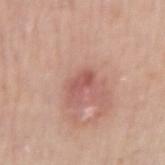Impression: No biopsy was performed on this lesion — it was imaged during a full skin examination and was not determined to be concerning. Image and clinical context: Longest diameter approximately 2.5 mm. The tile uses white-light illumination. Automated image analysis of the tile measured a lesion area of about 3 mm² and a symmetry-axis asymmetry near 0.35. It also reported a lesion color around L≈54 a*≈27 b*≈26 in CIELAB and about 9 CIELAB-L* units darker than the surrounding skin. Located on the right upper arm. A male subject, aged 58 to 62. Cropped from a whole-body photographic skin survey; the tile spans about 15 mm.